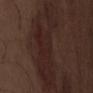The lesion was photographed on a routine skin check and not biopsied; there is no pathology result. Imaged with white-light lighting. The recorded lesion diameter is about 12 mm. This image is a 15 mm lesion crop taken from a total-body photograph. A male patient, approximately 70 years of age. Located on the abdomen.